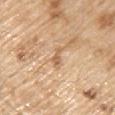Q: Lesion size?
A: ~3 mm (longest diameter)
Q: Where on the body is the lesion?
A: the left upper arm
Q: What are the patient's age and sex?
A: male, about 70 years old
Q: What is the imaging modality?
A: ~15 mm crop, total-body skin-cancer survey
Q: How was the tile lit?
A: white-light